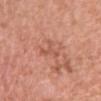Impression:
No biopsy was performed on this lesion — it was imaged during a full skin examination and was not determined to be concerning.
Acquisition and patient details:
This is a white-light tile. The lesion's longest dimension is about 3 mm. A 15 mm close-up tile from a total-body photography series done for melanoma screening. On the chest. A female patient aged 58 to 62.